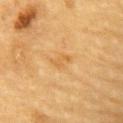Clinical summary:
The tile uses cross-polarized illumination. The lesion-visualizer software estimated an area of roughly 2.5 mm², a shape eccentricity near 0.9, and a symmetry-axis asymmetry near 0.45. The analysis additionally found a lesion color around L≈54 a*≈18 b*≈42 in CIELAB and roughly 6 lightness units darker than nearby skin. The analysis additionally found internal color variation of about 0 on a 0–10 scale and a peripheral color-asymmetry measure near 0. The software also gave an automated nevus-likeness rating near 0 out of 100 and a detector confidence of about 100 out of 100 that the crop contains a lesion. Measured at roughly 2.5 mm in maximum diameter. Cropped from a whole-body photographic skin survey; the tile spans about 15 mm. The lesion is on the left upper arm. A male patient about 85 years old or older.